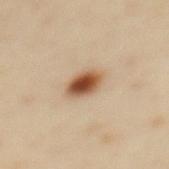biopsy_status: not biopsied; imaged during a skin examination
lighting: cross-polarized
automated_metrics:
  cielab_L: 54
  cielab_a: 21
  cielab_b: 34
  vs_skin_contrast_norm: 11.5
site: mid back
patient:
  sex: female
  age_approx: 40
image:
  source: total-body photography crop
  field_of_view_mm: 15
lesion_size:
  long_diameter_mm_approx: 3.5